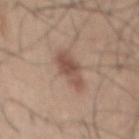site — the mid back | lesion size — about 4.5 mm | image — ~15 mm crop, total-body skin-cancer survey | subject — male, aged around 35 | lighting — white-light illumination.